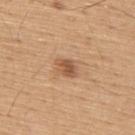{
  "biopsy_status": "not biopsied; imaged during a skin examination",
  "patient": {
    "sex": "male",
    "age_approx": 55
  },
  "lesion_size": {
    "long_diameter_mm_approx": 2.5
  },
  "site": "upper back",
  "image": {
    "source": "total-body photography crop",
    "field_of_view_mm": 15
  }
}